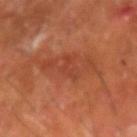Captured during whole-body skin photography for melanoma surveillance; the lesion was not biopsied. From the arm. A male subject aged around 60. Cropped from a whole-body photographic skin survey; the tile spans about 15 mm. About 3.5 mm across. Imaged with cross-polarized lighting.